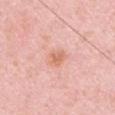workup: no biopsy performed (imaged during a skin exam)
automated metrics: an average lesion color of about L≈68 a*≈25 b*≈32 (CIELAB) and about 8 CIELAB-L* units darker than the surrounding skin; internal color variation of about 3 on a 0–10 scale
tile lighting: white-light illumination
anatomic site: the left upper arm
image source: 15 mm crop, total-body photography
diameter: ~2.5 mm (longest diameter)
subject: male, in their 50s